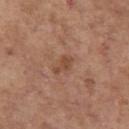Q: Was this lesion biopsied?
A: catalogued during a skin exam; not biopsied
Q: What is the imaging modality?
A: 15 mm crop, total-body photography
Q: Where on the body is the lesion?
A: the left upper arm
Q: What are the patient's age and sex?
A: female, in their mid-60s